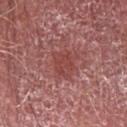Q: Is there a histopathology result?
A: imaged on a skin check; not biopsied
Q: Lesion size?
A: about 3 mm
Q: How was this image acquired?
A: 15 mm crop, total-body photography
Q: Where on the body is the lesion?
A: the right forearm
Q: What are the patient's age and sex?
A: male, approximately 70 years of age
Q: How was the tile lit?
A: white-light